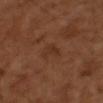<record>
<biopsy_status>not biopsied; imaged during a skin examination</biopsy_status>
<patient>
  <sex>male</sex>
  <age_approx>65</age_approx>
</patient>
<image>
  <source>total-body photography crop</source>
  <field_of_view_mm>15</field_of_view_mm>
</image>
</record>